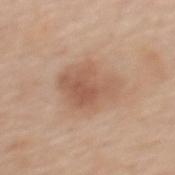No biopsy was performed on this lesion — it was imaged during a full skin examination and was not determined to be concerning. This is a white-light tile. Located on the upper back. The subject is a female aged 63–67. The lesion's longest dimension is about 5.5 mm. Automated image analysis of the tile measured a footprint of about 19 mm², a shape eccentricity near 0.65, and a shape-asymmetry score of about 0.25 (0 = symmetric). It also reported a nevus-likeness score of about 70/100. A roughly 15 mm field-of-view crop from a total-body skin photograph.